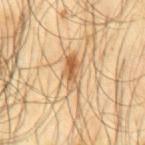{"biopsy_status": "not biopsied; imaged during a skin examination", "site": "mid back", "patient": {"sex": "male", "age_approx": 65}, "image": {"source": "total-body photography crop", "field_of_view_mm": 15}, "lighting": "cross-polarized"}